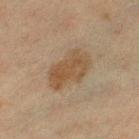Clinical impression: Recorded during total-body skin imaging; not selected for excision or biopsy. Clinical summary: From the left lower leg. The patient is a female aged approximately 40. The lesion's longest dimension is about 5.5 mm. This image is a 15 mm lesion crop taken from a total-body photograph.